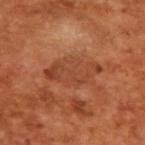Impression: This lesion was catalogued during total-body skin photography and was not selected for biopsy. Clinical summary: The recorded lesion diameter is about 6 mm. A male subject in their mid- to late 60s. Captured under cross-polarized illumination. A 15 mm close-up tile from a total-body photography series done for melanoma screening.